This image is a 15 mm lesion crop taken from a total-body photograph. The lesion is on the chest. A male patient, aged approximately 50. This is a cross-polarized tile.
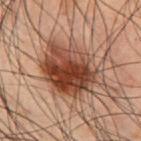Diagnosis:
Biopsy histopathology demonstrated a dysplastic (Clark) nevus.